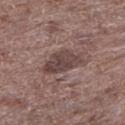Imaged during a routine full-body skin examination; the lesion was not biopsied and no histopathology is available.
Automated image analysis of the tile measured a lesion area of about 11 mm², an eccentricity of roughly 0.85, and a shape-asymmetry score of about 0.25 (0 = symmetric). And it measured a mean CIELAB color near L≈43 a*≈17 b*≈18 and a normalized lesion–skin contrast near 8.
Located on the left lower leg.
Imaged with white-light lighting.
A lesion tile, about 15 mm wide, cut from a 3D total-body photograph.
The recorded lesion diameter is about 5.5 mm.
A male patient aged around 70.